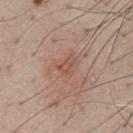biopsy status: total-body-photography surveillance lesion; no biopsy
site: the chest
lighting: white-light illumination
subject: male, aged approximately 55
image source: 15 mm crop, total-body photography